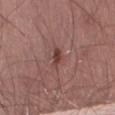The lesion was photographed on a routine skin check and not biopsied; there is no pathology result.
Located on the lower back.
The subject is a male approximately 60 years of age.
The lesion-visualizer software estimated a lesion area of about 2.5 mm² and a symmetry-axis asymmetry near 0.4. And it measured a lesion color around L≈41 a*≈22 b*≈23 in CIELAB and a normalized lesion–skin contrast near 8.
Measured at roughly 2.5 mm in maximum diameter.
This is a white-light tile.
A region of skin cropped from a whole-body photographic capture, roughly 15 mm wide.